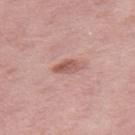follow-up=total-body-photography surveillance lesion; no biopsy
image source=~15 mm crop, total-body skin-cancer survey
anatomic site=the right thigh
lesion size=~3 mm (longest diameter)
patient=female, aged 38–42
automated metrics=a border-irregularity index near 1.5/10, internal color variation of about 4 on a 0–10 scale, and peripheral color asymmetry of about 1.5